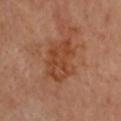Clinical impression:
This lesion was catalogued during total-body skin photography and was not selected for biopsy.
Image and clinical context:
A 15 mm close-up tile from a total-body photography series done for melanoma screening. On the head or neck. The recorded lesion diameter is about 5.5 mm. The subject is a male roughly 65 years of age.